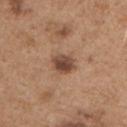The lesion was photographed on a routine skin check and not biopsied; there is no pathology result.
This is a white-light tile.
On the chest.
A lesion tile, about 15 mm wide, cut from a 3D total-body photograph.
Longest diameter approximately 3 mm.
An algorithmic analysis of the crop reported an area of roughly 6 mm², an outline eccentricity of about 0.6 (0 = round, 1 = elongated), and a shape-asymmetry score of about 0.2 (0 = symmetric). The software also gave an average lesion color of about L≈46 a*≈20 b*≈28 (CIELAB), a lesion–skin lightness drop of about 14, and a normalized border contrast of about 10. It also reported a border-irregularity index near 2/10, a color-variation rating of about 4/10, and a peripheral color-asymmetry measure near 1. And it measured an automated nevus-likeness rating near 80 out of 100 and a detector confidence of about 100 out of 100 that the crop contains a lesion.
A male subject, aged approximately 65.